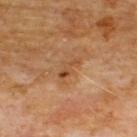<lesion>
<patient>
  <sex>male</sex>
  <age_approx>60</age_approx>
</patient>
<image>
  <source>total-body photography crop</source>
  <field_of_view_mm>15</field_of_view_mm>
</image>
<site>chest</site>
</lesion>